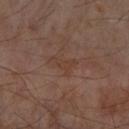This lesion was catalogued during total-body skin photography and was not selected for biopsy. The patient is a male aged around 65. The tile uses cross-polarized illumination. The lesion is on the arm. About 3.5 mm across. A roughly 15 mm field-of-view crop from a total-body skin photograph.